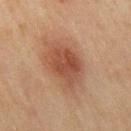Clinical impression:
The lesion was tiled from a total-body skin photograph and was not biopsied.
Context:
A female subject approximately 60 years of age. A lesion tile, about 15 mm wide, cut from a 3D total-body photograph. The lesion is located on the right thigh.